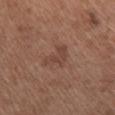Clinical impression:
This lesion was catalogued during total-body skin photography and was not selected for biopsy.
Acquisition and patient details:
On the upper back. The recorded lesion diameter is about 4 mm. A female subject, aged around 55. A roughly 15 mm field-of-view crop from a total-body skin photograph. The lesion-visualizer software estimated a nevus-likeness score of about 0/100. This is a white-light tile.